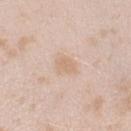follow-up: catalogued during a skin exam; not biopsied | diameter: ≈3 mm | automated lesion analysis: a nevus-likeness score of about 0/100 and a detector confidence of about 100 out of 100 that the crop contains a lesion | image: total-body-photography crop, ~15 mm field of view | body site: the arm | lighting: white-light illumination | patient: female, in their mid-20s.